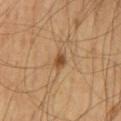Recorded during total-body skin imaging; not selected for excision or biopsy.
Captured under cross-polarized illumination.
A roughly 15 mm field-of-view crop from a total-body skin photograph.
Located on the mid back.
A male patient about 60 years old.